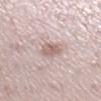Part of a total-body skin-imaging series; this lesion was reviewed on a skin check and was not flagged for biopsy. The lesion's longest dimension is about 2.5 mm. Located on the right lower leg. The tile uses white-light illumination. A female subject, roughly 40 years of age. A roughly 15 mm field-of-view crop from a total-body skin photograph.